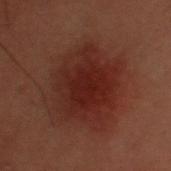– workup: imaged on a skin check; not biopsied
– size: ~7 mm (longest diameter)
– tile lighting: cross-polarized
– site: the head or neck
– image: ~15 mm crop, total-body skin-cancer survey
– patient: male, about 30 years old